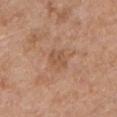Assessment: The lesion was tiled from a total-body skin photograph and was not biopsied. Background: On the left upper arm. A male subject aged approximately 65. The lesion's longest dimension is about 2.5 mm. This is a white-light tile. Cropped from a total-body skin-imaging series; the visible field is about 15 mm. An algorithmic analysis of the crop reported a footprint of about 4.5 mm², an eccentricity of roughly 0.7, and a shape-asymmetry score of about 0.25 (0 = symmetric). The analysis additionally found a mean CIELAB color near L≈53 a*≈21 b*≈32, a lesion–skin lightness drop of about 6, and a normalized lesion–skin contrast near 5. The analysis additionally found a classifier nevus-likeness of about 0/100.